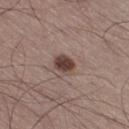Notes:
• follow-up · imaged on a skin check; not biopsied
• image · ~15 mm tile from a whole-body skin photo
• subject · male, aged approximately 55
• lesion diameter · about 2.5 mm
• lighting · white-light
• anatomic site · the right thigh